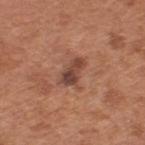Notes:
* follow-up · total-body-photography surveillance lesion; no biopsy
* acquisition · total-body-photography crop, ~15 mm field of view
* patient · male, aged 63 to 67
* anatomic site · the upper back
* lesion size · ≈3.5 mm
* lighting · white-light illumination